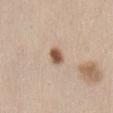{"biopsy_status": "not biopsied; imaged during a skin examination", "lesion_size": {"long_diameter_mm_approx": 2.5}, "image": {"source": "total-body photography crop", "field_of_view_mm": 15}, "patient": {"sex": "female", "age_approx": 40}, "site": "front of the torso", "lighting": "white-light"}